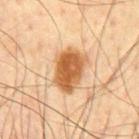Q: What is the lesion's diameter?
A: about 5 mm
Q: Lesion location?
A: the chest
Q: What lighting was used for the tile?
A: cross-polarized
Q: What kind of image is this?
A: total-body-photography crop, ~15 mm field of view
Q: Patient demographics?
A: male, aged 43 to 47